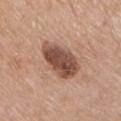  biopsy_status: not biopsied; imaged during a skin examination
  image:
    source: total-body photography crop
    field_of_view_mm: 15
  patient:
    sex: male
    age_approx: 80
  site: chest